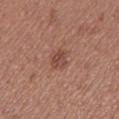On the left lower leg.
The tile uses white-light illumination.
The patient is a female aged 38 to 42.
Cropped from a whole-body photographic skin survey; the tile spans about 15 mm.
Measured at roughly 2.5 mm in maximum diameter.
The lesion-visualizer software estimated a mean CIELAB color near L≈47 a*≈23 b*≈26 and roughly 9 lightness units darker than nearby skin. The analysis additionally found a nevus-likeness score of about 25/100 and a detector confidence of about 100 out of 100 that the crop contains a lesion.